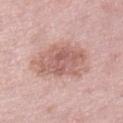{"biopsy_status": "not biopsied; imaged during a skin examination", "site": "left lower leg", "lesion_size": {"long_diameter_mm_approx": 7.0}, "automated_metrics": {"area_mm2_approx": 24.0, "eccentricity": 0.7, "shape_asymmetry": 0.25, "cielab_L": 61, "cielab_a": 22, "cielab_b": 24, "vs_skin_darker_L": 11.0, "vs_skin_contrast_norm": 7.0, "border_irregularity_0_10": 3.0, "color_variation_0_10": 4.5, "peripheral_color_asymmetry": 1.5, "nevus_likeness_0_100": 10, "lesion_detection_confidence_0_100": 100}, "patient": {"sex": "female", "age_approx": 40}, "image": {"source": "total-body photography crop", "field_of_view_mm": 15}}